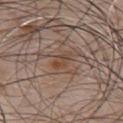biopsy status: total-body-photography surveillance lesion; no biopsy
anatomic site: the chest
patient: male, about 55 years old
imaging modality: ~15 mm crop, total-body skin-cancer survey